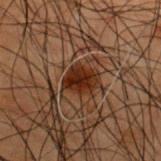site = the upper back
tile lighting = cross-polarized
lesion size = ≈3 mm
subject = male, aged approximately 50
imaging modality = 15 mm crop, total-body photography